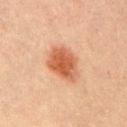Impression: Recorded during total-body skin imaging; not selected for excision or biopsy. Context: A 15 mm close-up tile from a total-body photography series done for melanoma screening. The lesion is on the mid back. A male patient about 70 years old.